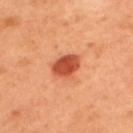Q: Was a biopsy performed?
A: no biopsy performed (imaged during a skin exam)
Q: What are the patient's age and sex?
A: male, aged 48–52
Q: What lighting was used for the tile?
A: cross-polarized
Q: What kind of image is this?
A: ~15 mm tile from a whole-body skin photo
Q: Lesion location?
A: the upper back
Q: How large is the lesion?
A: about 3.5 mm